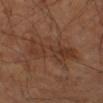{"biopsy_status": "not biopsied; imaged during a skin examination", "automated_metrics": {"area_mm2_approx": 16.0, "eccentricity": 0.95, "shape_asymmetry": 0.4, "border_irregularity_0_10": 6.5, "color_variation_0_10": 4.0, "peripheral_color_asymmetry": 1.5}, "site": "left forearm", "image": {"source": "total-body photography crop", "field_of_view_mm": 15}, "patient": {"sex": "male", "age_approx": 65}}